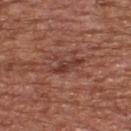  biopsy_status: not biopsied; imaged during a skin examination
  patient:
    sex: male
    age_approx: 65
  lesion_size:
    long_diameter_mm_approx: 4.0
  automated_metrics:
    eccentricity: 0.85
    nevus_likeness_0_100: 0
  image:
    source: total-body photography crop
    field_of_view_mm: 15
  lighting: white-light
  site: upper back